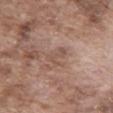Image and clinical context:
On the abdomen. A lesion tile, about 15 mm wide, cut from a 3D total-body photograph. A male patient, in their mid-70s.